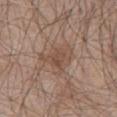workup: catalogued during a skin exam; not biopsied | anatomic site: the front of the torso | subject: male, about 65 years old | acquisition: total-body-photography crop, ~15 mm field of view.